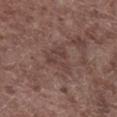* automated metrics — a mean CIELAB color near L≈40 a*≈18 b*≈21, about 6 CIELAB-L* units darker than the surrounding skin, and a normalized border contrast of about 5; a classifier nevus-likeness of about 0/100 and lesion-presence confidence of about 100/100
* lighting — white-light illumination
* location — the right lower leg
* acquisition — ~15 mm tile from a whole-body skin photo
* subject — male, aged around 70